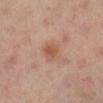workup = imaged on a skin check; not biopsied | anatomic site = the left leg | acquisition = 15 mm crop, total-body photography | patient = female, approximately 40 years of age.